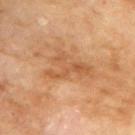The lesion was tiled from a total-body skin photograph and was not biopsied. Cropped from a total-body skin-imaging series; the visible field is about 15 mm. The patient is a male aged 68 to 72. The tile uses cross-polarized illumination. From the back. Measured at roughly 5.5 mm in maximum diameter.